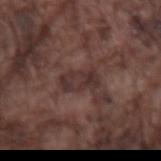| key | value |
|---|---|
| workup | total-body-photography surveillance lesion; no biopsy |
| patient | male, in their mid- to late 70s |
| anatomic site | the left forearm |
| image source | ~15 mm tile from a whole-body skin photo |
| diameter | ≈4 mm |
| automated metrics | a lesion area of about 7 mm², a shape eccentricity near 0.85, and two-axis asymmetry of about 0.2; about 7 CIELAB-L* units darker than the surrounding skin and a normalized border contrast of about 7.5; border irregularity of about 3 on a 0–10 scale, a color-variation rating of about 4/10, and radial color variation of about 1.5; a nevus-likeness score of about 0/100 |
| illumination | white-light illumination |